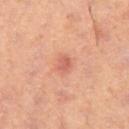Impression: This lesion was catalogued during total-body skin photography and was not selected for biopsy. Context: Located on the right thigh. A female patient, aged 38 to 42. A region of skin cropped from a whole-body photographic capture, roughly 15 mm wide. Longest diameter approximately 2 mm. The tile uses cross-polarized illumination. The total-body-photography lesion software estimated a lesion area of about 2.5 mm², a shape eccentricity near 0.55, and two-axis asymmetry of about 0.2. And it measured a border-irregularity index near 1.5/10, a within-lesion color-variation index near 3/10, and peripheral color asymmetry of about 1.5. The software also gave a classifier nevus-likeness of about 40/100 and lesion-presence confidence of about 100/100.